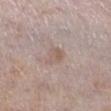{
  "biopsy_status": "not biopsied; imaged during a skin examination",
  "lesion_size": {
    "long_diameter_mm_approx": 3.0
  },
  "automated_metrics": {
    "cielab_L": 57,
    "cielab_a": 15,
    "cielab_b": 24,
    "vs_skin_darker_L": 6.0,
    "vs_skin_contrast_norm": 5.5
  },
  "image": {
    "source": "total-body photography crop",
    "field_of_view_mm": 15
  },
  "site": "right lower leg",
  "lighting": "white-light",
  "patient": {
    "sex": "male",
    "age_approx": 60
  }
}